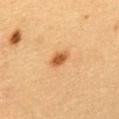{"biopsy_status": "not biopsied; imaged during a skin examination", "lighting": "cross-polarized", "site": "upper back", "automated_metrics": {"nevus_likeness_0_100": 100, "lesion_detection_confidence_0_100": 100}, "lesion_size": {"long_diameter_mm_approx": 2.5}, "patient": {"sex": "female", "age_approx": 30}, "image": {"source": "total-body photography crop", "field_of_view_mm": 15}}